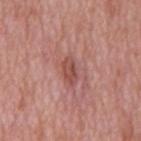Part of a total-body skin-imaging series; this lesion was reviewed on a skin check and was not flagged for biopsy. The patient is a male approximately 65 years of age. A lesion tile, about 15 mm wide, cut from a 3D total-body photograph. Captured under white-light illumination. From the mid back.